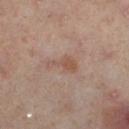Impression: This lesion was catalogued during total-body skin photography and was not selected for biopsy. Clinical summary: A 15 mm crop from a total-body photograph taken for skin-cancer surveillance. A female subject aged approximately 50. The lesion is located on the leg.